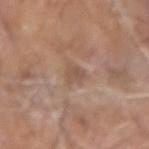Image and clinical context: The subject is a male aged 73–77. A 15 mm crop from a total-body photograph taken for skin-cancer surveillance. On the left forearm.